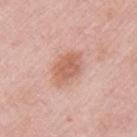Captured during whole-body skin photography for melanoma surveillance; the lesion was not biopsied.
A female subject, aged approximately 65.
A roughly 15 mm field-of-view crop from a total-body skin photograph.
On the left upper arm.